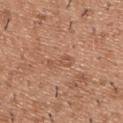Findings:
- workup: total-body-photography surveillance lesion; no biopsy
- image: 15 mm crop, total-body photography
- anatomic site: the upper back
- subject: male, aged approximately 40
- lesion size: about 3.5 mm
- lighting: white-light